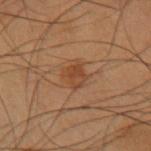Q: Was a biopsy performed?
A: total-body-photography surveillance lesion; no biopsy
Q: How large is the lesion?
A: ~3.5 mm (longest diameter)
Q: What kind of image is this?
A: ~15 mm crop, total-body skin-cancer survey
Q: Patient demographics?
A: male, roughly 55 years of age
Q: Lesion location?
A: the left upper arm
Q: Illumination type?
A: cross-polarized illumination